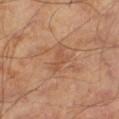<tbp_lesion>
  <biopsy_status>not biopsied; imaged during a skin examination</biopsy_status>
  <patient>
    <sex>male</sex>
    <age_approx>65</age_approx>
  </patient>
  <automated_metrics>
    <cielab_L>51</cielab_L>
    <cielab_a>21</cielab_a>
    <cielab_b>33</cielab_b>
    <vs_skin_darker_L>6.0</vs_skin_darker_L>
    <vs_skin_contrast_norm>5.0</vs_skin_contrast_norm>
    <border_irregularity_0_10>6.0</border_irregularity_0_10>
    <color_variation_0_10>2.0</color_variation_0_10>
    <peripheral_color_asymmetry>1.0</peripheral_color_asymmetry>
  </automated_metrics>
  <lesion_size>
    <long_diameter_mm_approx>4.5</long_diameter_mm_approx>
  </lesion_size>
  <image>
    <source>total-body photography crop</source>
    <field_of_view_mm>15</field_of_view_mm>
  </image>
  <lighting>cross-polarized</lighting>
</tbp_lesion>